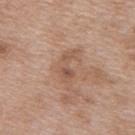workup: no biopsy performed (imaged during a skin exam)
tile lighting: white-light illumination
lesion size: ≈4 mm
body site: the upper back
image: total-body-photography crop, ~15 mm field of view
image-analysis metrics: a lesion area of about 5 mm², an outline eccentricity of about 0.9 (0 = round, 1 = elongated), and two-axis asymmetry of about 0.5; a lesion color around L≈54 a*≈20 b*≈29 in CIELAB, roughly 8 lightness units darker than nearby skin, and a normalized lesion–skin contrast near 6
patient: female, roughly 40 years of age